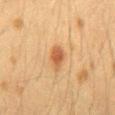{
  "biopsy_status": "not biopsied; imaged during a skin examination",
  "lighting": "cross-polarized",
  "patient": {
    "sex": "female",
    "age_approx": 40
  },
  "image": {
    "source": "total-body photography crop",
    "field_of_view_mm": 15
  },
  "site": "mid back"
}